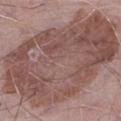Clinical impression:
Captured during whole-body skin photography for melanoma surveillance; the lesion was not biopsied.
Acquisition and patient details:
A 15 mm crop from a total-body photograph taken for skin-cancer surveillance. A male patient about 65 years old. On the leg. This is a white-light tile. Measured at roughly 16 mm in maximum diameter.